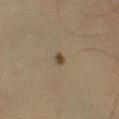Impression:
Recorded during total-body skin imaging; not selected for excision or biopsy.
Clinical summary:
Longest diameter approximately 1.5 mm. Located on the leg. This is a cross-polarized tile. A 15 mm close-up extracted from a 3D total-body photography capture. The subject is a male aged 38 to 42.